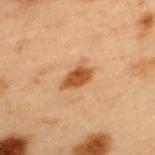Captured during whole-body skin photography for melanoma surveillance; the lesion was not biopsied.
This is a cross-polarized tile.
The lesion is on the back.
Measured at roughly 3.5 mm in maximum diameter.
A male subject, approximately 55 years of age.
A 15 mm close-up tile from a total-body photography series done for melanoma screening.